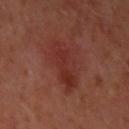biopsy status: no biopsy performed (imaged during a skin exam) | lighting: cross-polarized illumination | image-analysis metrics: a lesion area of about 15 mm² and a shape-asymmetry score of about 0.25 (0 = symmetric); a border-irregularity index near 4/10, a within-lesion color-variation index near 4/10, and radial color variation of about 1.5; a nevus-likeness score of about 0/100 and lesion-presence confidence of about 100/100 | patient: female, aged 38 to 42 | location: the left upper arm | imaging modality: total-body-photography crop, ~15 mm field of view | lesion size: ≈5.5 mm.